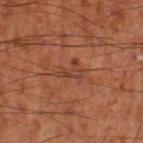Impression: Recorded during total-body skin imaging; not selected for excision or biopsy. Context: The patient is a male aged 53–57. Captured under cross-polarized illumination. This image is a 15 mm lesion crop taken from a total-body photograph. The lesion-visualizer software estimated a mean CIELAB color near L≈42 a*≈25 b*≈31, roughly 6 lightness units darker than nearby skin, and a normalized border contrast of about 5.5. And it measured border irregularity of about 8 on a 0–10 scale and a color-variation rating of about 0/10. It also reported an automated nevus-likeness rating near 0 out of 100 and a detector confidence of about 95 out of 100 that the crop contains a lesion. Located on the left thigh.